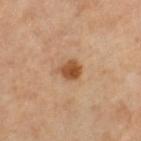Case summary:
* notes — catalogued during a skin exam; not biopsied
* anatomic site — the left thigh
* patient — female, roughly 55 years of age
* illumination — cross-polarized
* automated metrics — a lesion-detection confidence of about 100/100
* acquisition — ~15 mm crop, total-body skin-cancer survey
* lesion size — about 3 mm